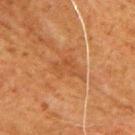Q: Was this lesion biopsied?
A: total-body-photography surveillance lesion; no biopsy
Q: What kind of image is this?
A: ~15 mm crop, total-body skin-cancer survey
Q: What did automated image analysis measure?
A: a border-irregularity rating of about 5/10, a color-variation rating of about 2/10, and peripheral color asymmetry of about 0.5; lesion-presence confidence of about 100/100
Q: Patient demographics?
A: male, approximately 80 years of age
Q: What is the anatomic site?
A: the arm
Q: How large is the lesion?
A: ~4 mm (longest diameter)